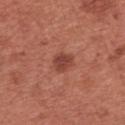Captured during whole-body skin photography for melanoma surveillance; the lesion was not biopsied.
Approximately 2.5 mm at its widest.
A female patient, in their 50s.
The lesion is located on the left forearm.
Automated image analysis of the tile measured a lesion area of about 4.5 mm², an outline eccentricity of about 0.5 (0 = round, 1 = elongated), and a symmetry-axis asymmetry near 0.25. The software also gave an average lesion color of about L≈43 a*≈27 b*≈28 (CIELAB), roughly 10 lightness units darker than nearby skin, and a normalized border contrast of about 8.
Cropped from a total-body skin-imaging series; the visible field is about 15 mm.